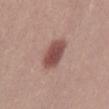Impression:
Part of a total-body skin-imaging series; this lesion was reviewed on a skin check and was not flagged for biopsy.
Image and clinical context:
The patient is a male roughly 30 years of age. The lesion-visualizer software estimated a footprint of about 8.5 mm², an eccentricity of roughly 0.8, and a symmetry-axis asymmetry near 0.1. The analysis additionally found an average lesion color of about L≈49 a*≈23 b*≈23 (CIELAB) and a lesion-to-skin contrast of about 10 (normalized; higher = more distinct). And it measured a border-irregularity rating of about 1/10 and a within-lesion color-variation index near 4/10. The lesion's longest dimension is about 4 mm. This image is a 15 mm lesion crop taken from a total-body photograph.